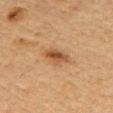<record>
<biopsy_status>not biopsied; imaged during a skin examination</biopsy_status>
<automated_metrics>
  <area_mm2_approx>5.5</area_mm2_approx>
  <eccentricity>0.8</eccentricity>
  <shape_asymmetry>0.3</shape_asymmetry>
  <vs_skin_darker_L>10.0</vs_skin_darker_L>
  <vs_skin_contrast_norm>8.0</vs_skin_contrast_norm>
  <border_irregularity_0_10>3.0</border_irregularity_0_10>
  <color_variation_0_10>4.5</color_variation_0_10>
  <peripheral_color_asymmetry>1.5</peripheral_color_asymmetry>
  <nevus_likeness_0_100>95</nevus_likeness_0_100>
</automated_metrics>
<lesion_size>
  <long_diameter_mm_approx>3.5</long_diameter_mm_approx>
</lesion_size>
<site>mid back</site>
<patient>
  <sex>female</sex>
  <age_approx>60</age_approx>
</patient>
<lighting>cross-polarized</lighting>
<image>
  <source>total-body photography crop</source>
  <field_of_view_mm>15</field_of_view_mm>
</image>
</record>